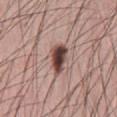Assessment:
No biopsy was performed on this lesion — it was imaged during a full skin examination and was not determined to be concerning.
Image and clinical context:
The subject is a male aged approximately 30. On the abdomen. Cropped from a whole-body photographic skin survey; the tile spans about 15 mm.